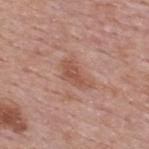Part of a total-body skin-imaging series; this lesion was reviewed on a skin check and was not flagged for biopsy. A 15 mm close-up tile from a total-body photography series done for melanoma screening. About 4 mm across. A male subject aged 53 to 57. The lesion is on the back.